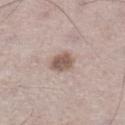This lesion was catalogued during total-body skin photography and was not selected for biopsy. From the left lower leg. A male patient, aged approximately 70. A lesion tile, about 15 mm wide, cut from a 3D total-body photograph. The lesion's longest dimension is about 3 mm. Captured under white-light illumination.